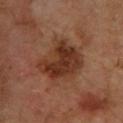| feature | finding |
|---|---|
| patient | male, approximately 65 years of age |
| anatomic site | the upper back |
| imaging modality | 15 mm crop, total-body photography |